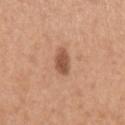The subject is a female in their mid-60s. The lesion's longest dimension is about 3 mm. The lesion is located on the left upper arm. A 15 mm close-up extracted from a 3D total-body photography capture.